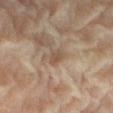biopsy_status: not biopsied; imaged during a skin examination
patient:
  sex: female
  age_approx: 80
image:
  source: total-body photography crop
  field_of_view_mm: 15
lighting: cross-polarized
automated_metrics:
  cielab_L: 43
  cielab_a: 13
  cielab_b: 24
  vs_skin_darker_L: 7.0
  vs_skin_contrast_norm: 6.0
site: right thigh
lesion_size:
  long_diameter_mm_approx: 3.0